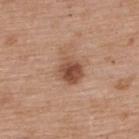Impression:
The lesion was tiled from a total-body skin photograph and was not biopsied.
Image and clinical context:
An algorithmic analysis of the crop reported a mean CIELAB color near L≈51 a*≈21 b*≈30, about 12 CIELAB-L* units darker than the surrounding skin, and a normalized lesion–skin contrast near 8.5. And it measured a border-irregularity index near 3/10 and a peripheral color-asymmetry measure near 2. The software also gave an automated nevus-likeness rating near 85 out of 100 and a detector confidence of about 100 out of 100 that the crop contains a lesion. Captured under white-light illumination. The lesion is located on the upper back. The subject is a female aged around 60. Cropped from a whole-body photographic skin survey; the tile spans about 15 mm.